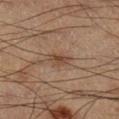Part of a total-body skin-imaging series; this lesion was reviewed on a skin check and was not flagged for biopsy. The lesion is on the leg. A male patient, aged approximately 60. Cropped from a total-body skin-imaging series; the visible field is about 15 mm.